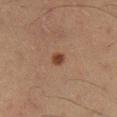Impression:
Captured during whole-body skin photography for melanoma surveillance; the lesion was not biopsied.